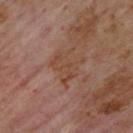biopsy status: total-body-photography surveillance lesion; no biopsy
location: the back
tile lighting: cross-polarized illumination
lesion diameter: ~4 mm (longest diameter)
acquisition: 15 mm crop, total-body photography
subject: male, in their 70s